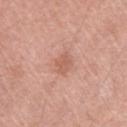This lesion was catalogued during total-body skin photography and was not selected for biopsy.
Captured under white-light illumination.
A 15 mm crop from a total-body photograph taken for skin-cancer surveillance.
Located on the right upper arm.
The patient is a male aged approximately 40.